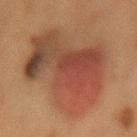Notes:
– notes: imaged on a skin check; not biopsied
– lesion size: about 13 mm
– tile lighting: cross-polarized
– site: the mid back
– image-analysis metrics: an area of roughly 65 mm², a shape eccentricity near 0.85, and a symmetry-axis asymmetry near 0.3; a lesion-to-skin contrast of about 8 (normalized; higher = more distinct); radial color variation of about 3.5; a lesion-detection confidence of about 100/100
– patient: female, roughly 50 years of age
– imaging modality: ~15 mm crop, total-body skin-cancer survey